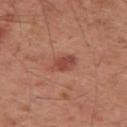Part of a total-body skin-imaging series; this lesion was reviewed on a skin check and was not flagged for biopsy. On the right upper arm. A region of skin cropped from a whole-body photographic capture, roughly 15 mm wide. An algorithmic analysis of the crop reported a mean CIELAB color near L≈47 a*≈27 b*≈28, about 10 CIELAB-L* units darker than the surrounding skin, and a lesion-to-skin contrast of about 7 (normalized; higher = more distinct). The analysis additionally found a color-variation rating of about 3/10. The software also gave a classifier nevus-likeness of about 75/100 and a detector confidence of about 100 out of 100 that the crop contains a lesion. A male patient, aged 58–62. Imaged with white-light lighting. Longest diameter approximately 3.5 mm.